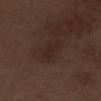Notes:
– notes · total-body-photography surveillance lesion; no biopsy